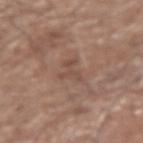The lesion was tiled from a total-body skin photograph and was not biopsied. This is a white-light tile. The lesion is located on the right forearm. A roughly 15 mm field-of-view crop from a total-body skin photograph. Longest diameter approximately 3 mm. A male patient in their mid-70s. An algorithmic analysis of the crop reported an area of roughly 4.5 mm² and an outline eccentricity of about 0.8 (0 = round, 1 = elongated). The software also gave a lesion color around L≈48 a*≈18 b*≈25 in CIELAB and a lesion–skin lightness drop of about 6. The analysis additionally found border irregularity of about 7.5 on a 0–10 scale, a within-lesion color-variation index near 0/10, and a peripheral color-asymmetry measure near 0. The software also gave a nevus-likeness score of about 0/100.